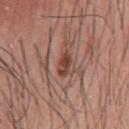No biopsy was performed on this lesion — it was imaged during a full skin examination and was not determined to be concerning.